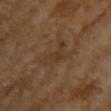Recorded during total-body skin imaging; not selected for excision or biopsy.
A 15 mm close-up extracted from a 3D total-body photography capture.
The lesion is located on the chest.
The tile uses cross-polarized illumination.
Measured at roughly 5 mm in maximum diameter.
A female patient approximately 70 years of age.
Automated tile analysis of the lesion measured a footprint of about 7.5 mm², an outline eccentricity of about 0.9 (0 = round, 1 = elongated), and a shape-asymmetry score of about 0.55 (0 = symmetric). It also reported lesion-presence confidence of about 70/100.